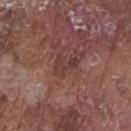| key | value |
|---|---|
| illumination | white-light |
| image | ~15 mm crop, total-body skin-cancer survey |
| size | about 3 mm |
| subject | male, aged around 75 |
| anatomic site | the right forearm |